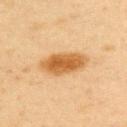This lesion was catalogued during total-body skin photography and was not selected for biopsy.
A lesion tile, about 15 mm wide, cut from a 3D total-body photograph.
About 5.5 mm across.
A female patient, roughly 45 years of age.
Located on the back.
Captured under cross-polarized illumination.